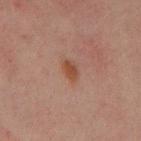| key | value |
|---|---|
| notes | total-body-photography surveillance lesion; no biopsy |
| image | ~15 mm crop, total-body skin-cancer survey |
| tile lighting | cross-polarized illumination |
| diameter | ≈2.5 mm |
| automated lesion analysis | an average lesion color of about L≈38 a*≈19 b*≈26 (CIELAB), a lesion–skin lightness drop of about 7, and a normalized lesion–skin contrast near 7.5 |
| subject | male, about 30 years old |
| anatomic site | the mid back |